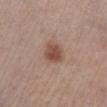{"biopsy_status": "not biopsied; imaged during a skin examination", "automated_metrics": {"area_mm2_approx": 6.0, "eccentricity": 0.4, "nevus_likeness_0_100": 95, "lesion_detection_confidence_0_100": 100}, "site": "right lower leg", "lighting": "white-light", "patient": {"sex": "male", "age_approx": 60}, "lesion_size": {"long_diameter_mm_approx": 2.5}, "image": {"source": "total-body photography crop", "field_of_view_mm": 15}}